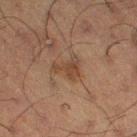notes: catalogued during a skin exam; not biopsied | lesion diameter: ~3 mm (longest diameter) | anatomic site: the right thigh | patient: male, aged 58–62 | imaging modality: ~15 mm crop, total-body skin-cancer survey.